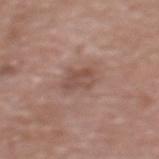* follow-up — imaged on a skin check; not biopsied
* subject — female, aged approximately 70
* anatomic site — the upper back
* illumination — white-light illumination
* diameter — ≈3.5 mm
* TBP lesion metrics — a footprint of about 5.5 mm², an outline eccentricity of about 0.75 (0 = round, 1 = elongated), and a symmetry-axis asymmetry near 0.25; a border-irregularity rating of about 3/10, a color-variation rating of about 3/10, and radial color variation of about 1
* acquisition — 15 mm crop, total-body photography